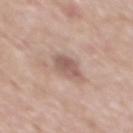notes: no biopsy performed (imaged during a skin exam) | imaging modality: 15 mm crop, total-body photography | diameter: about 3 mm | subject: male, about 60 years old | automated lesion analysis: a lesion area of about 5.5 mm², a shape eccentricity near 0.7, and two-axis asymmetry of about 0.25; an average lesion color of about L≈56 a*≈18 b*≈23 (CIELAB), about 11 CIELAB-L* units darker than the surrounding skin, and a lesion-to-skin contrast of about 7 (normalized; higher = more distinct); a nevus-likeness score of about 5/100 and lesion-presence confidence of about 100/100 | tile lighting: white-light | body site: the mid back.